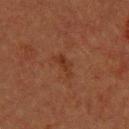patient = male, aged 38–42
acquisition = ~15 mm tile from a whole-body skin photo
location = the upper back
automated lesion analysis = two-axis asymmetry of about 0.45; an automated nevus-likeness rating near 10 out of 100 and a detector confidence of about 100 out of 100 that the crop contains a lesion
tile lighting = cross-polarized